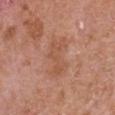This is a white-light tile. Longest diameter approximately 4.5 mm. A male subject in their mid- to late 60s. Located on the chest. Cropped from a whole-body photographic skin survey; the tile spans about 15 mm.